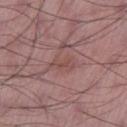{
  "biopsy_status": "not biopsied; imaged during a skin examination",
  "site": "right thigh",
  "lesion_size": {
    "long_diameter_mm_approx": 3.5
  },
  "image": {
    "source": "total-body photography crop",
    "field_of_view_mm": 15
  },
  "patient": {
    "sex": "male",
    "age_approx": 50
  }
}